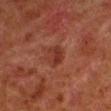Imaged during a routine full-body skin examination; the lesion was not biopsied and no histopathology is available.
Captured under cross-polarized illumination.
A male patient, aged 78 to 82.
The lesion is on the left lower leg.
About 2.5 mm across.
An algorithmic analysis of the crop reported an eccentricity of roughly 0.7 and a shape-asymmetry score of about 0.25 (0 = symmetric). And it measured internal color variation of about 3 on a 0–10 scale and a peripheral color-asymmetry measure near 1. The analysis additionally found a nevus-likeness score of about 50/100 and a lesion-detection confidence of about 100/100.
A 15 mm close-up tile from a total-body photography series done for melanoma screening.